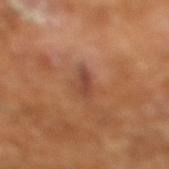Clinical impression:
Imaged during a routine full-body skin examination; the lesion was not biopsied and no histopathology is available.
Background:
The tile uses cross-polarized illumination. Measured at roughly 3 mm in maximum diameter. The patient is a male roughly 65 years of age. A lesion tile, about 15 mm wide, cut from a 3D total-body photograph.